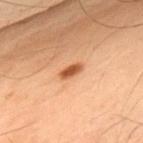On the upper back. A 15 mm crop from a total-body photograph taken for skin-cancer surveillance. The subject is a male aged 48–52. The lesion's longest dimension is about 3 mm. Captured under cross-polarized illumination.